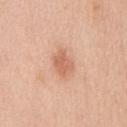<record>
  <biopsy_status>not biopsied; imaged during a skin examination</biopsy_status>
  <patient>
    <sex>male</sex>
    <age_approx>50</age_approx>
  </patient>
  <site>chest</site>
  <image>
    <source>total-body photography crop</source>
    <field_of_view_mm>15</field_of_view_mm>
  </image>
</record>